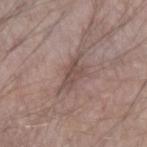notes: catalogued during a skin exam; not biopsied
anatomic site: the left forearm
acquisition: 15 mm crop, total-body photography
subject: male, roughly 70 years of age